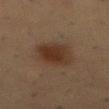Part of a total-body skin-imaging series; this lesion was reviewed on a skin check and was not flagged for biopsy. From the mid back. The lesion's longest dimension is about 4.5 mm. The patient is a male approximately 55 years of age. Cropped from a total-body skin-imaging series; the visible field is about 15 mm. Imaged with cross-polarized lighting.